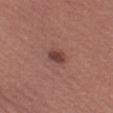Q: Was this lesion biopsied?
A: imaged on a skin check; not biopsied
Q: Lesion location?
A: the left thigh
Q: What is the imaging modality?
A: ~15 mm tile from a whole-body skin photo
Q: What is the lesion's diameter?
A: about 2.5 mm
Q: What lighting was used for the tile?
A: white-light
Q: Patient demographics?
A: female, aged around 60